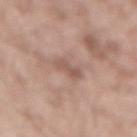workup: no biopsy performed (imaged during a skin exam); subject: male, aged 53 to 57; lesion diameter: about 2.5 mm; location: the lower back; imaging modality: total-body-photography crop, ~15 mm field of view.